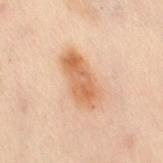A female subject aged 48–52.
The total-body-photography lesion software estimated an automated nevus-likeness rating near 80 out of 100.
The lesion is on the leg.
Measured at roughly 6 mm in maximum diameter.
A 15 mm crop from a total-body photograph taken for skin-cancer surveillance.